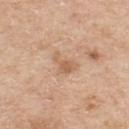{"biopsy_status": "not biopsied; imaged during a skin examination", "site": "back", "lesion_size": {"long_diameter_mm_approx": 3.0}, "image": {"source": "total-body photography crop", "field_of_view_mm": 15}, "lighting": "white-light", "patient": {"sex": "male", "age_approx": 60}, "automated_metrics": {"area_mm2_approx": 4.5, "eccentricity": 0.75, "shape_asymmetry": 0.6, "cielab_L": 61, "cielab_a": 19, "cielab_b": 34, "vs_skin_contrast_norm": 5.5, "color_variation_0_10": 1.5, "peripheral_color_asymmetry": 0.5}}